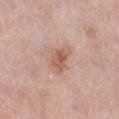follow-up: imaged on a skin check; not biopsied | size: ≈3 mm | image source: total-body-photography crop, ~15 mm field of view | subject: female, aged 38 to 42 | automated lesion analysis: a lesion area of about 5.5 mm² and a shape-asymmetry score of about 0.25 (0 = symmetric); a border-irregularity rating of about 2.5/10, a color-variation rating of about 3/10, and peripheral color asymmetry of about 1; a classifier nevus-likeness of about 45/100 and a lesion-detection confidence of about 100/100 | body site: the leg | tile lighting: white-light illumination.